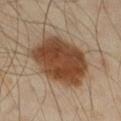Q: Was this lesion biopsied?
A: no biopsy performed (imaged during a skin exam)
Q: What lighting was used for the tile?
A: cross-polarized illumination
Q: How large is the lesion?
A: ~7 mm (longest diameter)
Q: Automated lesion metrics?
A: an area of roughly 33 mm² and two-axis asymmetry of about 0.15; a nevus-likeness score of about 100/100 and a lesion-detection confidence of about 100/100
Q: What are the patient's age and sex?
A: male, approximately 45 years of age
Q: Lesion location?
A: the left thigh
Q: How was this image acquired?
A: total-body-photography crop, ~15 mm field of view